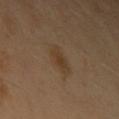<tbp_lesion>
<biopsy_status>not biopsied; imaged during a skin examination</biopsy_status>
<site>left upper arm</site>
<lesion_size>
  <long_diameter_mm_approx>3.5</long_diameter_mm_approx>
</lesion_size>
<patient>
  <sex>male</sex>
  <age_approx>40</age_approx>
</patient>
<image>
  <source>total-body photography crop</source>
  <field_of_view_mm>15</field_of_view_mm>
</image>
</tbp_lesion>